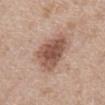Clinical impression:
This lesion was catalogued during total-body skin photography and was not selected for biopsy.
Clinical summary:
A male patient about 65 years old. The tile uses white-light illumination. This image is a 15 mm lesion crop taken from a total-body photograph. On the chest. Automated image analysis of the tile measured an average lesion color of about L≈54 a*≈20 b*≈27 (CIELAB), a lesion–skin lightness drop of about 13, and a normalized lesion–skin contrast near 9. Measured at roughly 6.5 mm in maximum diameter.